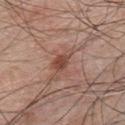  biopsy_status: not biopsied; imaged during a skin examination
  lesion_size:
    long_diameter_mm_approx: 3.5
  image:
    source: total-body photography crop
    field_of_view_mm: 15
  patient:
    sex: male
    age_approx: 50
  site: chest